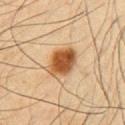• workup — total-body-photography surveillance lesion; no biopsy
• subject — male, aged approximately 65
• lesion diameter — ~4 mm (longest diameter)
• imaging modality — ~15 mm crop, total-body skin-cancer survey
• illumination — cross-polarized illumination
• site — the chest